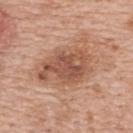Clinical summary: The subject is a female aged 58–62. A 15 mm close-up extracted from a 3D total-body photography capture. Located on the upper back.